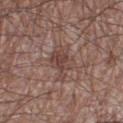Impression: The lesion was photographed on a routine skin check and not biopsied; there is no pathology result. Image and clinical context: The total-body-photography lesion software estimated a footprint of about 9 mm², a shape eccentricity near 0.9, and two-axis asymmetry of about 0.35. It also reported a classifier nevus-likeness of about 0/100 and a detector confidence of about 90 out of 100 that the crop contains a lesion. A region of skin cropped from a whole-body photographic capture, roughly 15 mm wide. A male patient in their 60s. Measured at roughly 5.5 mm in maximum diameter. From the leg.